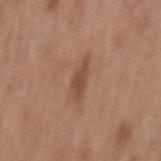notes — no biopsy performed (imaged during a skin exam)
imaging modality — total-body-photography crop, ~15 mm field of view
lesion size — ~4 mm (longest diameter)
location — the mid back
subject — male, aged approximately 60
TBP lesion metrics — about 9 CIELAB-L* units darker than the surrounding skin and a normalized border contrast of about 6.5; a border-irregularity index near 4/10, internal color variation of about 1 on a 0–10 scale, and peripheral color asymmetry of about 0.5